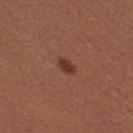{
  "biopsy_status": "not biopsied; imaged during a skin examination",
  "site": "right thigh",
  "image": {
    "source": "total-body photography crop",
    "field_of_view_mm": 15
  },
  "lesion_size": {
    "long_diameter_mm_approx": 2.5
  },
  "patient": {
    "sex": "female",
    "age_approx": 25
  },
  "lighting": "white-light",
  "automated_metrics": {
    "cielab_L": 36,
    "cielab_a": 23,
    "cielab_b": 25,
    "vs_skin_darker_L": 10.0,
    "vs_skin_contrast_norm": 8.5
  }
}